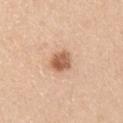Impression:
This lesion was catalogued during total-body skin photography and was not selected for biopsy.
Clinical summary:
A 15 mm crop from a total-body photograph taken for skin-cancer surveillance. From the right upper arm. The subject is a male roughly 30 years of age. The tile uses white-light illumination. Automated image analysis of the tile measured an area of roughly 5.5 mm², an eccentricity of roughly 0.5, and two-axis asymmetry of about 0.2. And it measured a mean CIELAB color near L≈59 a*≈22 b*≈34 and a normalized lesion–skin contrast near 9. The analysis additionally found a border-irregularity rating of about 2/10 and a color-variation rating of about 4/10.